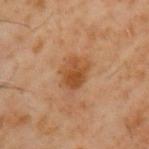– image: total-body-photography crop, ~15 mm field of view
– image-analysis metrics: a shape-asymmetry score of about 0.2 (0 = symmetric); lesion-presence confidence of about 100/100
– lighting: cross-polarized
– subject: male, about 60 years old
– body site: the left upper arm